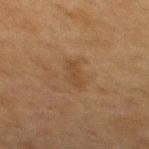This lesion was catalogued during total-body skin photography and was not selected for biopsy.
A male subject roughly 75 years of age.
A 15 mm crop from a total-body photograph taken for skin-cancer surveillance.
Located on the mid back.